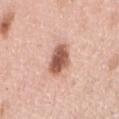Imaged during a routine full-body skin examination; the lesion was not biopsied and no histopathology is available.
The tile uses white-light illumination.
The lesion's longest dimension is about 3.5 mm.
This image is a 15 mm lesion crop taken from a total-body photograph.
The total-body-photography lesion software estimated a footprint of about 9 mm², an eccentricity of roughly 0.55, and a shape-asymmetry score of about 0.15 (0 = symmetric).
A female patient, aged 48–52.
The lesion is located on the arm.